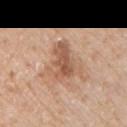notes: total-body-photography surveillance lesion; no biopsy
TBP lesion metrics: a lesion color around L≈57 a*≈20 b*≈32 in CIELAB and roughly 10 lightness units darker than nearby skin; a border-irregularity rating of about 5.5/10, a within-lesion color-variation index near 4.5/10, and peripheral color asymmetry of about 1.5; an automated nevus-likeness rating near 0 out of 100 and a lesion-detection confidence of about 100/100
lighting: white-light illumination
body site: the left upper arm
image: total-body-photography crop, ~15 mm field of view
patient: male, roughly 65 years of age
lesion size: ≈5 mm